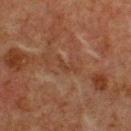No biopsy was performed on this lesion — it was imaged during a full skin examination and was not determined to be concerning.
The patient is a male in their mid- to late 70s.
This is a cross-polarized tile.
A 15 mm close-up extracted from a 3D total-body photography capture.
On the chest.